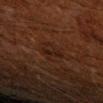<case>
<biopsy_status>not biopsied; imaged during a skin examination</biopsy_status>
<image>
  <source>total-body photography crop</source>
  <field_of_view_mm>15</field_of_view_mm>
</image>
<site>arm</site>
<patient>
  <sex>male</sex>
  <age_approx>60</age_approx>
</patient>
</case>